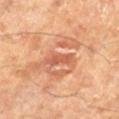Clinical summary:
A male subject, aged 68–72. The lesion is on the left lower leg. This is a cross-polarized tile. A 15 mm close-up tile from a total-body photography series done for melanoma screening.